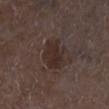Assessment: The lesion was photographed on a routine skin check and not biopsied; there is no pathology result. Context: The lesion is on the right lower leg. A 15 mm crop from a total-body photograph taken for skin-cancer surveillance. The subject is a male in their mid-70s.